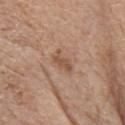<record>
  <biopsy_status>not biopsied; imaged during a skin examination</biopsy_status>
</record>